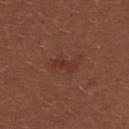Captured during whole-body skin photography for melanoma surveillance; the lesion was not biopsied. A female patient in their mid-20s. The lesion is on the upper back. A close-up tile cropped from a whole-body skin photograph, about 15 mm across.